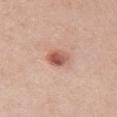Imaged during a routine full-body skin examination; the lesion was not biopsied and no histopathology is available. A female patient, aged around 40. Longest diameter approximately 3.5 mm. The total-body-photography lesion software estimated an area of roughly 6 mm² and a symmetry-axis asymmetry near 0.2. The analysis additionally found a mean CIELAB color near L≈58 a*≈24 b*≈28, a lesion–skin lightness drop of about 13, and a normalized lesion–skin contrast near 8.5. The analysis additionally found a border-irregularity rating of about 2/10, a color-variation rating of about 7/10, and a peripheral color-asymmetry measure near 2.5. This image is a 15 mm lesion crop taken from a total-body photograph. Located on the front of the torso. The tile uses white-light illumination.